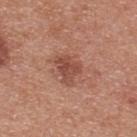{
  "biopsy_status": "not biopsied; imaged during a skin examination",
  "site": "back",
  "lesion_size": {
    "long_diameter_mm_approx": 3.5
  },
  "patient": {
    "sex": "male",
    "age_approx": 30
  },
  "image": {
    "source": "total-body photography crop",
    "field_of_view_mm": 15
  }
}